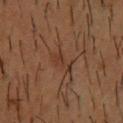The lesion was photographed on a routine skin check and not biopsied; there is no pathology result.
The recorded lesion diameter is about 3.5 mm.
A close-up tile cropped from a whole-body skin photograph, about 15 mm across.
On the chest.
The tile uses cross-polarized illumination.
A male patient, in their mid-50s.